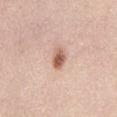Part of a total-body skin-imaging series; this lesion was reviewed on a skin check and was not flagged for biopsy. The total-body-photography lesion software estimated a footprint of about 4.5 mm², a shape eccentricity near 0.75, and a symmetry-axis asymmetry near 0.2. It also reported a lesion color around L≈60 a*≈21 b*≈30 in CIELAB, a lesion–skin lightness drop of about 15, and a normalized lesion–skin contrast near 9.5. The analysis additionally found border irregularity of about 1.5 on a 0–10 scale and a peripheral color-asymmetry measure near 1.5. The analysis additionally found a classifier nevus-likeness of about 95/100 and a lesion-detection confidence of about 100/100. The patient is a female aged approximately 55. A region of skin cropped from a whole-body photographic capture, roughly 15 mm wide. The lesion is located on the lower back. The recorded lesion diameter is about 3 mm.